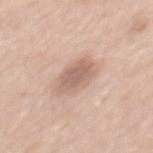Findings:
– follow-up · no biopsy performed (imaged during a skin exam)
– acquisition · total-body-photography crop, ~15 mm field of view
– illumination · white-light
– site · the back
– automated lesion analysis · an outline eccentricity of about 0.8 (0 = round, 1 = elongated) and a shape-asymmetry score of about 0.15 (0 = symmetric); a lesion color around L≈63 a*≈18 b*≈27 in CIELAB and a normalized lesion–skin contrast near 7; a border-irregularity rating of about 2/10, a within-lesion color-variation index near 3/10, and a peripheral color-asymmetry measure near 1; a lesion-detection confidence of about 100/100
– subject · male, aged around 50
– lesion size · ~5 mm (longest diameter)